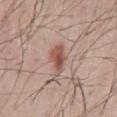notes: total-body-photography surveillance lesion; no biopsy | anatomic site: the abdomen | imaging modality: ~15 mm tile from a whole-body skin photo | subject: male, about 60 years old | lighting: white-light illumination | image-analysis metrics: a footprint of about 6 mm² and two-axis asymmetry of about 0.35; a lesion color around L≈53 a*≈22 b*≈25 in CIELAB and a normalized border contrast of about 8; a border-irregularity rating of about 3.5/10, a within-lesion color-variation index near 4/10, and peripheral color asymmetry of about 1.